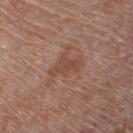Impression:
The lesion was tiled from a total-body skin photograph and was not biopsied.
Background:
The patient is a male aged 78–82. About 3.5 mm across. A 15 mm close-up tile from a total-body photography series done for melanoma screening. The lesion is on the front of the torso. The tile uses white-light illumination. An algorithmic analysis of the crop reported a border-irregularity rating of about 5/10, internal color variation of about 1.5 on a 0–10 scale, and a peripheral color-asymmetry measure near 0.5.